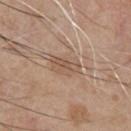Captured during whole-body skin photography for melanoma surveillance; the lesion was not biopsied.
The recorded lesion diameter is about 3.5 mm.
A 15 mm close-up tile from a total-body photography series done for melanoma screening.
On the chest.
A male patient in their mid- to late 60s.
This is a white-light tile.